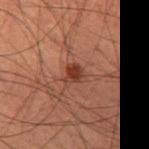<record>
  <biopsy_status>not biopsied; imaged during a skin examination</biopsy_status>
  <patient>
    <sex>male</sex>
    <age_approx>60</age_approx>
  </patient>
  <lighting>cross-polarized</lighting>
  <image>
    <source>total-body photography crop</source>
    <field_of_view_mm>15</field_of_view_mm>
  </image>
  <site>left thigh</site>
</record>